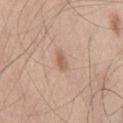Recorded during total-body skin imaging; not selected for excision or biopsy.
Cropped from a total-body skin-imaging series; the visible field is about 15 mm.
The lesion's longest dimension is about 2.5 mm.
On the right thigh.
The subject is a male roughly 45 years of age.
The lesion-visualizer software estimated a lesion area of about 3 mm² and an outline eccentricity of about 0.9 (0 = round, 1 = elongated). The analysis additionally found roughly 9 lightness units darker than nearby skin. It also reported a border-irregularity rating of about 4/10, a color-variation rating of about 1.5/10, and peripheral color asymmetry of about 0.5.
The tile uses white-light illumination.